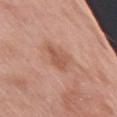The lesion was photographed on a routine skin check and not biopsied; there is no pathology result.
A male subject about 65 years old.
The lesion is on the right upper arm.
A 15 mm close-up tile from a total-body photography series done for melanoma screening.
This is a white-light tile.
The total-body-photography lesion software estimated a mean CIELAB color near L≈56 a*≈23 b*≈31, roughly 8 lightness units darker than nearby skin, and a lesion-to-skin contrast of about 6 (normalized; higher = more distinct). It also reported an automated nevus-likeness rating near 0 out of 100 and a lesion-detection confidence of about 100/100.